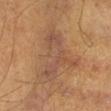Case summary:
- biopsy status: no biopsy performed (imaged during a skin exam)
- lighting: cross-polarized
- imaging modality: ~15 mm crop, total-body skin-cancer survey
- site: the left leg
- subject: male, approximately 55 years of age
- image-analysis metrics: a footprint of about 19 mm² and a symmetry-axis asymmetry near 0.55; a lesion–skin lightness drop of about 6 and a lesion-to-skin contrast of about 6 (normalized; higher = more distinct); a nevus-likeness score of about 0/100 and a lesion-detection confidence of about 75/100
- size: ≈7 mm